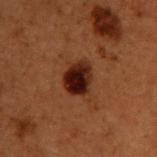biopsy status: imaged on a skin check; not biopsied
location: the upper back
lighting: cross-polarized
image source: total-body-photography crop, ~15 mm field of view
size: about 3.5 mm
TBP lesion metrics: an eccentricity of roughly 0.4 and a shape-asymmetry score of about 0.2 (0 = symmetric); a border-irregularity rating of about 2/10, a color-variation rating of about 6/10, and radial color variation of about 1.5; a nevus-likeness score of about 95/100 and a lesion-detection confidence of about 100/100
patient: male, aged approximately 50